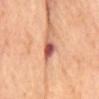On the mid back. About 3 mm across. A roughly 15 mm field-of-view crop from a total-body skin photograph. Captured under cross-polarized illumination.